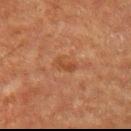{"biopsy_status": "not biopsied; imaged during a skin examination", "lighting": "cross-polarized", "image": {"source": "total-body photography crop", "field_of_view_mm": 15}, "site": "left upper arm", "lesion_size": {"long_diameter_mm_approx": 2.5}, "patient": {"sex": "male", "age_approx": 75}}